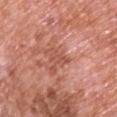workup — catalogued during a skin exam; not biopsied
lighting — white-light
image — ~15 mm tile from a whole-body skin photo
patient — male, aged 68–72
lesion diameter — about 3 mm
location — the upper back